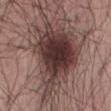Q: Is there a histopathology result?
A: catalogued during a skin exam; not biopsied
Q: What is the anatomic site?
A: the abdomen
Q: Who is the patient?
A: male, aged around 55
Q: How was the tile lit?
A: white-light illumination
Q: How was this image acquired?
A: ~15 mm tile from a whole-body skin photo